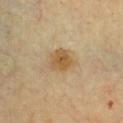Q: Is there a histopathology result?
A: imaged on a skin check; not biopsied
Q: What lighting was used for the tile?
A: cross-polarized
Q: Patient demographics?
A: male, in their mid-50s
Q: Lesion size?
A: ≈3.5 mm
Q: Automated lesion metrics?
A: an outline eccentricity of about 0.55 (0 = round, 1 = elongated) and a symmetry-axis asymmetry near 0.2; a lesion color around L≈57 a*≈15 b*≈39 in CIELAB and a normalized lesion–skin contrast near 8; internal color variation of about 3.5 on a 0–10 scale and a peripheral color-asymmetry measure near 1
Q: What kind of image is this?
A: ~15 mm crop, total-body skin-cancer survey
Q: Lesion location?
A: the chest